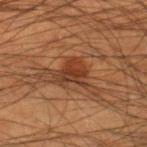workup: no biopsy performed (imaged during a skin exam) | diameter: about 3.5 mm | patient: male, aged around 45 | tile lighting: cross-polarized illumination | acquisition: ~15 mm tile from a whole-body skin photo | site: the right lower leg.